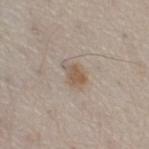* follow-up: catalogued during a skin exam; not biopsied
* site: the right thigh
* illumination: white-light
* automated metrics: a lesion color around L≈55 a*≈13 b*≈26 in CIELAB, a lesion–skin lightness drop of about 9, and a normalized lesion–skin contrast near 7; an automated nevus-likeness rating near 70 out of 100
* subject: female, in their 60s
* imaging modality: 15 mm crop, total-body photography
* lesion size: ≈3 mm